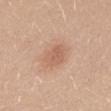workup: catalogued during a skin exam; not biopsied | site: the mid back | imaging modality: total-body-photography crop, ~15 mm field of view | TBP lesion metrics: a lesion color around L≈60 a*≈22 b*≈31 in CIELAB, roughly 8 lightness units darker than nearby skin, and a normalized lesion–skin contrast near 5; an automated nevus-likeness rating near 35 out of 100 and a detector confidence of about 100 out of 100 that the crop contains a lesion | tile lighting: white-light illumination | subject: female, aged approximately 25.